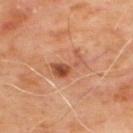The lesion was tiled from a total-body skin photograph and was not biopsied. About 4 mm across. An algorithmic analysis of the crop reported a footprint of about 7 mm² and a shape eccentricity near 0.85. A male subject, aged approximately 65. Captured under cross-polarized illumination. The lesion is on the upper back. A 15 mm close-up extracted from a 3D total-body photography capture.